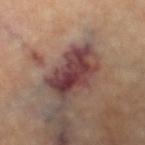Captured during whole-body skin photography for melanoma surveillance; the lesion was not biopsied.
This is a cross-polarized tile.
Automated tile analysis of the lesion measured a lesion color around L≈40 a*≈23 b*≈19 in CIELAB. It also reported a lesion-detection confidence of about 100/100.
The lesion is on the right lower leg.
A female patient aged approximately 65.
A close-up tile cropped from a whole-body skin photograph, about 15 mm across.
About 6 mm across.